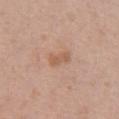Imaged during a routine full-body skin examination; the lesion was not biopsied and no histopathology is available.
Automated tile analysis of the lesion measured a mean CIELAB color near L≈58 a*≈20 b*≈31, a lesion–skin lightness drop of about 8, and a normalized border contrast of about 6. The software also gave a border-irregularity rating of about 2.5/10 and radial color variation of about 0. The analysis additionally found a classifier nevus-likeness of about 0/100.
A female patient in their 40s.
Imaged with white-light lighting.
Located on the chest.
A lesion tile, about 15 mm wide, cut from a 3D total-body photograph.